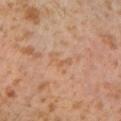A lesion tile, about 15 mm wide, cut from a 3D total-body photograph.
Longest diameter approximately 3 mm.
Captured under cross-polarized illumination.
The patient is a male aged 28–32.
The total-body-photography lesion software estimated a lesion area of about 3 mm².
On the left lower leg.